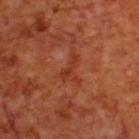Impression:
No biopsy was performed on this lesion — it was imaged during a full skin examination and was not determined to be concerning.
Acquisition and patient details:
A male subject aged 68–72. The lesion is on the back. A lesion tile, about 15 mm wide, cut from a 3D total-body photograph.